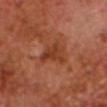Q: Was a biopsy performed?
A: imaged on a skin check; not biopsied
Q: How was the tile lit?
A: cross-polarized
Q: Patient demographics?
A: male, roughly 65 years of age
Q: How large is the lesion?
A: ≈3.5 mm
Q: What kind of image is this?
A: 15 mm crop, total-body photography